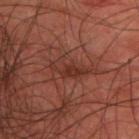biopsy status: catalogued during a skin exam; not biopsied | location: the upper back | image: total-body-photography crop, ~15 mm field of view | illumination: cross-polarized | subject: male, roughly 45 years of age | diameter: ≈5.5 mm.